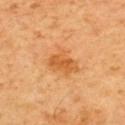Assessment: This lesion was catalogued during total-body skin photography and was not selected for biopsy. Background: A male subject, aged 58 to 62. Located on the upper back. A lesion tile, about 15 mm wide, cut from a 3D total-body photograph. Automated image analysis of the tile measured a footprint of about 12 mm². Measured at roughly 5 mm in maximum diameter.